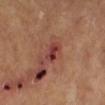lighting — cross-polarized
imaging modality — ~15 mm crop, total-body skin-cancer survey
site — the left leg
automated lesion analysis — a footprint of about 4 mm², a shape eccentricity near 0.8, and a shape-asymmetry score of about 0.35 (0 = symmetric); border irregularity of about 3 on a 0–10 scale and radial color variation of about 1.5; an automated nevus-likeness rating near 0 out of 100 and lesion-presence confidence of about 100/100
diameter — about 2.5 mm
patient — male, about 65 years old